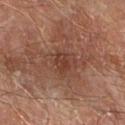A 15 mm close-up extracted from a 3D total-body photography capture.
This is a cross-polarized tile.
The lesion is on the right forearm.
About 2.5 mm across.
Automated image analysis of the tile measured a shape eccentricity near 0.65 and a shape-asymmetry score of about 0.25 (0 = symmetric). The analysis additionally found a nevus-likeness score of about 0/100.
The subject is a male aged approximately 70.